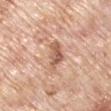No biopsy was performed on this lesion — it was imaged during a full skin examination and was not determined to be concerning. A lesion tile, about 15 mm wide, cut from a 3D total-body photograph. The lesion is on the left upper arm. A male subject, approximately 70 years of age. The recorded lesion diameter is about 4.5 mm.